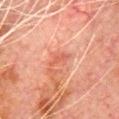Imaged during a routine full-body skin examination; the lesion was not biopsied and no histopathology is available. On the chest. The tile uses cross-polarized illumination. A 15 mm crop from a total-body photograph taken for skin-cancer surveillance. Measured at roughly 3 mm in maximum diameter. A male patient aged 78 to 82. Automated tile analysis of the lesion measured a footprint of about 3 mm², an eccentricity of roughly 0.95, and two-axis asymmetry of about 0.4. It also reported a mean CIELAB color near L≈47 a*≈26 b*≈28 and roughly 7 lightness units darker than nearby skin. The analysis additionally found a nevus-likeness score of about 0/100 and a detector confidence of about 100 out of 100 that the crop contains a lesion.